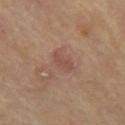Recorded during total-body skin imaging; not selected for excision or biopsy. Located on the mid back. A male subject about 70 years old. Automated image analysis of the tile measured a shape eccentricity near 0.8 and two-axis asymmetry of about 0.3. It also reported an automated nevus-likeness rating near 5 out of 100. Captured under cross-polarized illumination. A lesion tile, about 15 mm wide, cut from a 3D total-body photograph. About 2.5 mm across.